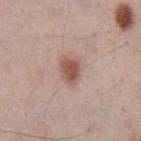Case summary:
* biopsy status — no biopsy performed (imaged during a skin exam)
* site — the abdomen
* acquisition — ~15 mm tile from a whole-body skin photo
* patient — male, aged 53 to 57
* lighting — white-light
* diameter — ~3 mm (longest diameter)